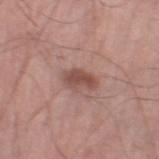Imaged during a routine full-body skin examination; the lesion was not biopsied and no histopathology is available.
Automated tile analysis of the lesion measured a lesion area of about 5 mm², an eccentricity of roughly 0.85, and a symmetry-axis asymmetry near 0.3. The analysis additionally found a border-irregularity index near 3/10, a color-variation rating of about 2/10, and radial color variation of about 0.5. The software also gave a nevus-likeness score of about 70/100 and lesion-presence confidence of about 100/100.
The patient is a male in their 40s.
Located on the left lower leg.
A 15 mm crop from a total-body photograph taken for skin-cancer surveillance.
This is a white-light tile.